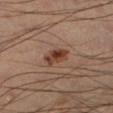Q: Is there a histopathology result?
A: catalogued during a skin exam; not biopsied
Q: What is the anatomic site?
A: the left lower leg
Q: What kind of image is this?
A: 15 mm crop, total-body photography
Q: What are the patient's age and sex?
A: male, about 45 years old
Q: What lighting was used for the tile?
A: cross-polarized illumination
Q: Automated lesion metrics?
A: a classifier nevus-likeness of about 95/100